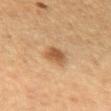This lesion was catalogued during total-body skin photography and was not selected for biopsy. The subject is a female aged around 40. The recorded lesion diameter is about 3 mm. Imaged with cross-polarized lighting. The lesion is located on the left forearm. A region of skin cropped from a whole-body photographic capture, roughly 15 mm wide. An algorithmic analysis of the crop reported a lesion area of about 6 mm² and an eccentricity of roughly 0.65. And it measured roughly 10 lightness units darker than nearby skin and a lesion-to-skin contrast of about 7.5 (normalized; higher = more distinct). The software also gave a border-irregularity index near 2/10, internal color variation of about 2.5 on a 0–10 scale, and a peripheral color-asymmetry measure near 1. The software also gave a classifier nevus-likeness of about 85/100 and a lesion-detection confidence of about 100/100.